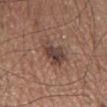The lesion was photographed on a routine skin check and not biopsied; there is no pathology result. The lesion's longest dimension is about 4.5 mm. A female patient, roughly 65 years of age. The lesion-visualizer software estimated an average lesion color of about L≈42 a*≈17 b*≈22 (CIELAB), roughly 10 lightness units darker than nearby skin, and a lesion-to-skin contrast of about 8.5 (normalized; higher = more distinct). The analysis additionally found border irregularity of about 3.5 on a 0–10 scale and internal color variation of about 6 on a 0–10 scale. It also reported an automated nevus-likeness rating near 65 out of 100 and a detector confidence of about 100 out of 100 that the crop contains a lesion. A 15 mm close-up extracted from a 3D total-body photography capture. The tile uses white-light illumination. The lesion is located on the head or neck.